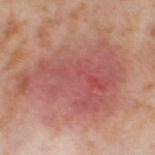Impression: Imaged during a routine full-body skin examination; the lesion was not biopsied and no histopathology is available. Context: A 15 mm close-up extracted from a 3D total-body photography capture. Automated image analysis of the tile measured a mean CIELAB color near L≈54 a*≈28 b*≈25, about 9 CIELAB-L* units darker than the surrounding skin, and a normalized border contrast of about 6.5. It also reported a border-irregularity index near 5.5/10, a color-variation rating of about 6/10, and peripheral color asymmetry of about 2. The analysis additionally found a classifier nevus-likeness of about 0/100 and lesion-presence confidence of about 95/100. Imaged with cross-polarized lighting. The lesion's longest dimension is about 11 mm. On the right thigh. The patient is a female aged approximately 55.